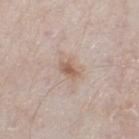Captured during whole-body skin photography for melanoma surveillance; the lesion was not biopsied.
Captured under white-light illumination.
Automated image analysis of the tile measured an area of roughly 4.5 mm², an outline eccentricity of about 0.75 (0 = round, 1 = elongated), and two-axis asymmetry of about 0.25. The analysis additionally found a border-irregularity rating of about 2.5/10, a color-variation rating of about 3/10, and peripheral color asymmetry of about 1.
The lesion is located on the right lower leg.
The recorded lesion diameter is about 3 mm.
A male patient, aged around 80.
A roughly 15 mm field-of-view crop from a total-body skin photograph.